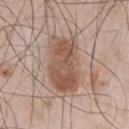| field | value |
|---|---|
| follow-up | no biopsy performed (imaged during a skin exam) |
| patient | male, approximately 55 years of age |
| image | total-body-photography crop, ~15 mm field of view |
| diameter | ≈7 mm |
| lighting | white-light illumination |
| automated metrics | a lesion area of about 21 mm², an outline eccentricity of about 0.85 (0 = round, 1 = elongated), and a shape-asymmetry score of about 0.2 (0 = symmetric); a lesion color around L≈53 a*≈18 b*≈28 in CIELAB, roughly 11 lightness units darker than nearby skin, and a normalized lesion–skin contrast near 8; a border-irregularity index near 3/10 and peripheral color asymmetry of about 1; a classifier nevus-likeness of about 100/100 |
| anatomic site | the chest |